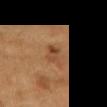No biopsy was performed on this lesion — it was imaged during a full skin examination and was not determined to be concerning. The tile uses cross-polarized illumination. An algorithmic analysis of the crop reported a lesion area of about 3 mm² and a shape eccentricity near 0.9. The analysis additionally found a lesion color around L≈40 a*≈20 b*≈32 in CIELAB, a lesion–skin lightness drop of about 9, and a normalized lesion–skin contrast near 7. The software also gave a color-variation rating of about 0.5/10 and a peripheral color-asymmetry measure near 0. It also reported an automated nevus-likeness rating near 30 out of 100 and a lesion-detection confidence of about 100/100. A 15 mm close-up extracted from a 3D total-body photography capture. The lesion is located on the mid back. A male patient, approximately 55 years of age.